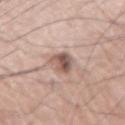Q: Is there a histopathology result?
A: catalogued during a skin exam; not biopsied
Q: What kind of image is this?
A: ~15 mm crop, total-body skin-cancer survey
Q: Lesion location?
A: the arm
Q: What are the patient's age and sex?
A: male, aged 73–77